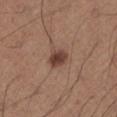notes: no biopsy performed (imaged during a skin exam) | image source: ~15 mm crop, total-body skin-cancer survey | site: the left lower leg | patient: male, approximately 65 years of age.